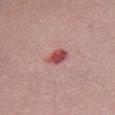The patient is a female aged approximately 65.
Longest diameter approximately 3 mm.
The lesion-visualizer software estimated a border-irregularity rating of about 2.5/10, a color-variation rating of about 3/10, and a peripheral color-asymmetry measure near 1. It also reported an automated nevus-likeness rating near 0 out of 100 and lesion-presence confidence of about 100/100.
A 15 mm close-up extracted from a 3D total-body photography capture.
The tile uses white-light illumination.
Located on the mid back.